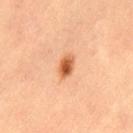Q: Was a biopsy performed?
A: total-body-photography surveillance lesion; no biopsy
Q: How was the tile lit?
A: cross-polarized
Q: Who is the patient?
A: female, in their 50s
Q: What is the imaging modality?
A: total-body-photography crop, ~15 mm field of view
Q: Automated lesion metrics?
A: a lesion area of about 4 mm² and a shape eccentricity near 0.8; border irregularity of about 1.5 on a 0–10 scale and internal color variation of about 5.5 on a 0–10 scale
Q: Lesion size?
A: ~2.5 mm (longest diameter)